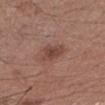The lesion was tiled from a total-body skin photograph and was not biopsied. Imaged with white-light lighting. A lesion tile, about 15 mm wide, cut from a 3D total-body photograph. A female subject, roughly 55 years of age. Located on the right forearm.